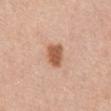Impression:
No biopsy was performed on this lesion — it was imaged during a full skin examination and was not determined to be concerning.
Context:
This is a white-light tile. Longest diameter approximately 3.5 mm. This image is a 15 mm lesion crop taken from a total-body photograph. The subject is a male in their 70s. The lesion-visualizer software estimated a lesion area of about 6.5 mm², a shape eccentricity near 0.65, and a shape-asymmetry score of about 0.2 (0 = symmetric). And it measured a within-lesion color-variation index near 2/10 and radial color variation of about 0.5. On the abdomen.